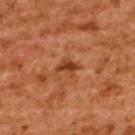The lesion was photographed on a routine skin check and not biopsied; there is no pathology result.
The patient is a female about 55 years old.
Cropped from a whole-body photographic skin survey; the tile spans about 15 mm.
Automated image analysis of the tile measured a mean CIELAB color near L≈44 a*≈30 b*≈40, roughly 11 lightness units darker than nearby skin, and a lesion-to-skin contrast of about 8 (normalized; higher = more distinct). The analysis additionally found a border-irregularity rating of about 3.5/10, a within-lesion color-variation index near 2.5/10, and radial color variation of about 0.5.
Located on the back.
About 2.5 mm across.
Imaged with cross-polarized lighting.